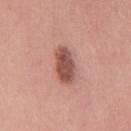Imaged during a routine full-body skin examination; the lesion was not biopsied and no histopathology is available.
This is a white-light tile.
The subject is a male about 40 years old.
Automated tile analysis of the lesion measured a mean CIELAB color near L≈52 a*≈24 b*≈26 and about 15 CIELAB-L* units darker than the surrounding skin. The software also gave a color-variation rating of about 3.5/10 and radial color variation of about 1. And it measured a classifier nevus-likeness of about 90/100 and lesion-presence confidence of about 100/100.
On the back.
Measured at roughly 4.5 mm in maximum diameter.
Cropped from a total-body skin-imaging series; the visible field is about 15 mm.